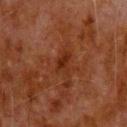| field | value |
|---|---|
| illumination | cross-polarized illumination |
| location | the upper back |
| automated metrics | an outline eccentricity of about 0.8 (0 = round, 1 = elongated) and two-axis asymmetry of about 0.35; a border-irregularity index near 3.5/10, a color-variation rating of about 2.5/10, and radial color variation of about 1; lesion-presence confidence of about 100/100 |
| patient | male, roughly 80 years of age |
| acquisition | ~15 mm tile from a whole-body skin photo |